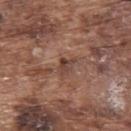No biopsy was performed on this lesion — it was imaged during a full skin examination and was not determined to be concerning. Approximately 2.5 mm at its widest. A 15 mm close-up extracted from a 3D total-body photography capture. A male patient, roughly 75 years of age. Captured under white-light illumination. On the upper back.